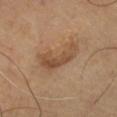Imaged during a routine full-body skin examination; the lesion was not biopsied and no histopathology is available. The lesion is on the leg. Cropped from a whole-body photographic skin survey; the tile spans about 15 mm. A subject aged 63–67. The lesion's longest dimension is about 5 mm. This is a cross-polarized tile.